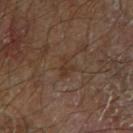{
  "site": "left forearm",
  "lesion_size": {
    "long_diameter_mm_approx": 2.5
  },
  "patient": {
    "sex": "male",
    "age_approx": 70
  },
  "lighting": "cross-polarized",
  "automated_metrics": {
    "area_mm2_approx": 4.0,
    "eccentricity": 0.65,
    "shape_asymmetry": 0.35,
    "lesion_detection_confidence_0_100": 100
  },
  "image": {
    "source": "total-body photography crop",
    "field_of_view_mm": 15
  }
}